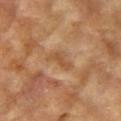Q: Was this lesion biopsied?
A: imaged on a skin check; not biopsied
Q: What lighting was used for the tile?
A: cross-polarized illumination
Q: Who is the patient?
A: female, roughly 75 years of age
Q: How was this image acquired?
A: 15 mm crop, total-body photography
Q: What is the lesion's diameter?
A: about 3.5 mm
Q: Lesion location?
A: the left upper arm
Q: What did automated image analysis measure?
A: a footprint of about 5 mm² and a shape eccentricity near 0.85; an automated nevus-likeness rating near 0 out of 100 and a lesion-detection confidence of about 95/100